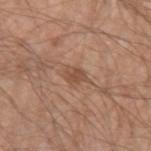Recorded during total-body skin imaging; not selected for excision or biopsy. A region of skin cropped from a whole-body photographic capture, roughly 15 mm wide. Imaged with white-light lighting. Longest diameter approximately 2.5 mm. A male subject aged approximately 65. On the left upper arm.